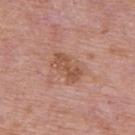Q: Is there a histopathology result?
A: catalogued during a skin exam; not biopsied
Q: How was this image acquired?
A: ~15 mm crop, total-body skin-cancer survey
Q: Where on the body is the lesion?
A: the upper back
Q: Lesion size?
A: about 4 mm
Q: What are the patient's age and sex?
A: male, in their mid-70s
Q: How was the tile lit?
A: white-light illumination
Q: What did automated image analysis measure?
A: a mean CIELAB color near L≈53 a*≈22 b*≈30, a lesion–skin lightness drop of about 8, and a normalized border contrast of about 6.5; a border-irregularity index near 3/10, a color-variation rating of about 2.5/10, and peripheral color asymmetry of about 1